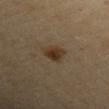{
  "patient": {
    "sex": "male",
    "age_approx": 65
  },
  "image": {
    "source": "total-body photography crop",
    "field_of_view_mm": 15
  },
  "site": "left upper arm",
  "automated_metrics": {
    "eccentricity": 0.6,
    "shape_asymmetry": 0.3
  },
  "lighting": "cross-polarized"
}